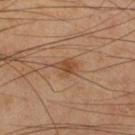Impression: This lesion was catalogued during total-body skin photography and was not selected for biopsy. Image and clinical context: Longest diameter approximately 2.5 mm. The tile uses cross-polarized illumination. From the right thigh. A 15 mm close-up tile from a total-body photography series done for melanoma screening. A male patient, aged approximately 70. Automated tile analysis of the lesion measured border irregularity of about 3.5 on a 0–10 scale and a peripheral color-asymmetry measure near 0.5. The analysis additionally found an automated nevus-likeness rating near 80 out of 100 and a detector confidence of about 100 out of 100 that the crop contains a lesion.